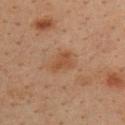| key | value |
|---|---|
| workup | no biopsy performed (imaged during a skin exam) |
| body site | the back |
| lighting | cross-polarized |
| image | 15 mm crop, total-body photography |
| subject | male, approximately 35 years of age |
| size | ≈3 mm |